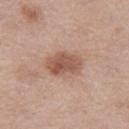Clinical impression: The lesion was tiled from a total-body skin photograph and was not biopsied. Clinical summary: A 15 mm crop from a total-body photograph taken for skin-cancer surveillance. Imaged with white-light lighting. From the leg. A female patient, aged around 40. The lesion-visualizer software estimated a nevus-likeness score of about 50/100 and a lesion-detection confidence of about 100/100. Approximately 4 mm at its widest.